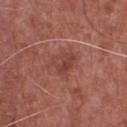The lesion was photographed on a routine skin check and not biopsied; there is no pathology result.
Located on the chest.
Imaged with white-light lighting.
A 15 mm crop from a total-body photograph taken for skin-cancer surveillance.
A male patient approximately 75 years of age.
The recorded lesion diameter is about 3 mm.